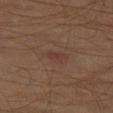Q: Is there a histopathology result?
A: no biopsy performed (imaged during a skin exam)
Q: Patient demographics?
A: male, aged 58 to 62
Q: What kind of image is this?
A: 15 mm crop, total-body photography
Q: What did automated image analysis measure?
A: a footprint of about 4.5 mm² and a symmetry-axis asymmetry near 0.2
Q: Where on the body is the lesion?
A: the left thigh
Q: How was the tile lit?
A: cross-polarized illumination